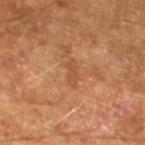Notes:
* workup — imaged on a skin check; not biopsied
* image — ~15 mm tile from a whole-body skin photo
* patient — male, roughly 65 years of age